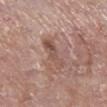No biopsy was performed on this lesion — it was imaged during a full skin examination and was not determined to be concerning. Longest diameter approximately 4.5 mm. A female subject about 75 years old. From the right lower leg. The tile uses white-light illumination. Cropped from a whole-body photographic skin survey; the tile spans about 15 mm. The lesion-visualizer software estimated a footprint of about 7 mm², a shape eccentricity near 0.9, and a symmetry-axis asymmetry near 0.35. And it measured a nevus-likeness score of about 0/100 and a lesion-detection confidence of about 100/100.